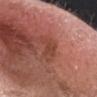The lesion was photographed on a routine skin check and not biopsied; there is no pathology result.
A male patient, aged 73–77.
From the head or neck.
A 15 mm crop from a total-body photograph taken for skin-cancer surveillance.